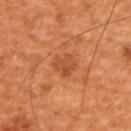The lesion was tiled from a total-body skin photograph and was not biopsied. About 3 mm across. The lesion-visualizer software estimated a lesion area of about 4 mm² and a shape eccentricity near 0.65. From the upper back. A male patient aged 63–67. Imaged with cross-polarized lighting. Cropped from a total-body skin-imaging series; the visible field is about 15 mm.